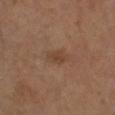Imaged during a routine full-body skin examination; the lesion was not biopsied and no histopathology is available.
A female subject aged approximately 65.
On the leg.
A 15 mm close-up tile from a total-body photography series done for melanoma screening.